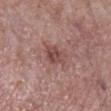Q: Was a biopsy performed?
A: total-body-photography surveillance lesion; no biopsy
Q: What are the patient's age and sex?
A: female, approximately 70 years of age
Q: Illumination type?
A: white-light
Q: What is the imaging modality?
A: total-body-photography crop, ~15 mm field of view
Q: What did automated image analysis measure?
A: radial color variation of about 1
Q: How large is the lesion?
A: ~3.5 mm (longest diameter)
Q: Lesion location?
A: the right lower leg